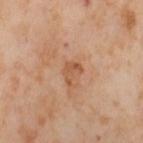No biopsy was performed on this lesion — it was imaged during a full skin examination and was not determined to be concerning.
A 15 mm close-up extracted from a 3D total-body photography capture.
The lesion is on the leg.
A female subject, in their mid- to late 50s.
Captured under cross-polarized illumination.